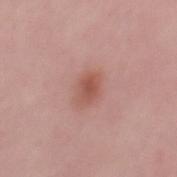The lesion was photographed on a routine skin check and not biopsied; there is no pathology result.
The lesion-visualizer software estimated a lesion area of about 5 mm² and a shape eccentricity near 0.75. It also reported a mean CIELAB color near L≈53 a*≈25 b*≈27, roughly 9 lightness units darker than nearby skin, and a normalized border contrast of about 7. It also reported a color-variation rating of about 3/10 and radial color variation of about 1. And it measured a nevus-likeness score of about 90/100 and lesion-presence confidence of about 100/100.
Captured under white-light illumination.
A 15 mm crop from a total-body photograph taken for skin-cancer surveillance.
From the mid back.
A male subject, roughly 30 years of age.